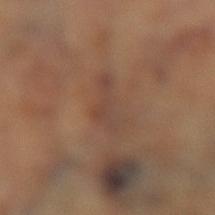biopsy_status: not biopsied; imaged during a skin examination
lighting: cross-polarized
site: left lower leg
automated_metrics:
  shape_asymmetry: 0.55
  border_irregularity_0_10: 6.5
  color_variation_0_10: 2.5
  nevus_likeness_0_100: 0
  lesion_detection_confidence_0_100: 85
lesion_size:
  long_diameter_mm_approx: 5.0
image:
  source: total-body photography crop
  field_of_view_mm: 15